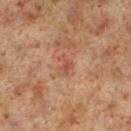Case summary:
- notes — no biopsy performed (imaged during a skin exam)
- lesion size — ≈2.5 mm
- subject — male, aged approximately 60
- location — the left lower leg
- imaging modality — ~15 mm crop, total-body skin-cancer survey